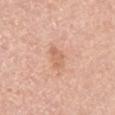{"lesion_size": {"long_diameter_mm_approx": 2.5}, "image": {"source": "total-body photography crop", "field_of_view_mm": 15}, "patient": {"sex": "female", "age_approx": 65}, "lighting": "white-light", "site": "right lower leg"}